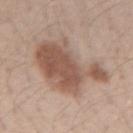Clinical impression: Part of a total-body skin-imaging series; this lesion was reviewed on a skin check and was not flagged for biopsy. Clinical summary: The lesion's longest dimension is about 9 mm. Imaged with white-light lighting. A female patient, aged 38 to 42. On the left forearm. A lesion tile, about 15 mm wide, cut from a 3D total-body photograph. Automated tile analysis of the lesion measured a classifier nevus-likeness of about 80/100 and a lesion-detection confidence of about 100/100.